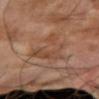Case summary:
• biopsy status · no biopsy performed (imaged during a skin exam)
• tile lighting · cross-polarized illumination
• automated lesion analysis · a mean CIELAB color near L≈45 a*≈20 b*≈29, a lesion–skin lightness drop of about 7, and a normalized lesion–skin contrast near 5.5; a detector confidence of about 85 out of 100 that the crop contains a lesion
• patient · male, approximately 70 years of age
• lesion size · ≈6.5 mm
• site · the right upper arm
• imaging modality · ~15 mm crop, total-body skin-cancer survey